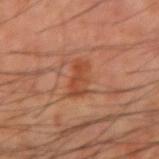Captured during whole-body skin photography for melanoma surveillance; the lesion was not biopsied.
A male subject aged 58 to 62.
The lesion is on the left forearm.
A roughly 15 mm field-of-view crop from a total-body skin photograph.
Measured at roughly 4 mm in maximum diameter.
Imaged with cross-polarized lighting.